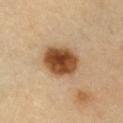Findings:
– follow-up: total-body-photography surveillance lesion; no biopsy
– acquisition: total-body-photography crop, ~15 mm field of view
– patient: female, aged 58 to 62
– lesion size: about 4.5 mm
– site: the chest